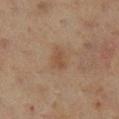Impression: Recorded during total-body skin imaging; not selected for excision or biopsy. Context: A female patient, aged around 55. A 15 mm close-up extracted from a 3D total-body photography capture. On the right lower leg. This is a cross-polarized tile. About 2.5 mm across.